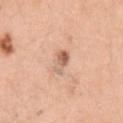{
  "biopsy_status": "not biopsied; imaged during a skin examination",
  "site": "left upper arm",
  "patient": {
    "sex": "female",
    "age_approx": 45
  },
  "image": {
    "source": "total-body photography crop",
    "field_of_view_mm": 15
  }
}